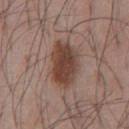  biopsy_status: not biopsied; imaged during a skin examination
  automated_metrics:
    area_mm2_approx: 15.0
    eccentricity: 0.6
    shape_asymmetry: 0.2
    vs_skin_contrast_norm: 10.0
    nevus_likeness_0_100: 90
    lesion_detection_confidence_0_100: 100
  lesion_size:
    long_diameter_mm_approx: 5.0
  patient:
    sex: male
    age_approx: 55
  site: front of the torso
  lighting: white-light
  image:
    source: total-body photography crop
    field_of_view_mm: 15